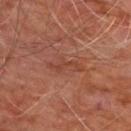Impression:
The lesion was photographed on a routine skin check and not biopsied; there is no pathology result.
Acquisition and patient details:
A 15 mm close-up extracted from a 3D total-body photography capture. The lesion is on the chest. The recorded lesion diameter is about 4 mm. A male subject aged approximately 60. This is a cross-polarized tile.